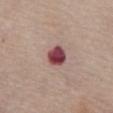Clinical impression: Part of a total-body skin-imaging series; this lesion was reviewed on a skin check and was not flagged for biopsy. Acquisition and patient details: An algorithmic analysis of the crop reported an area of roughly 5.5 mm² and an eccentricity of roughly 0.2. And it measured an average lesion color of about L≈44 a*≈28 b*≈18 (CIELAB), a lesion–skin lightness drop of about 19, and a lesion-to-skin contrast of about 14 (normalized; higher = more distinct). The analysis additionally found a border-irregularity rating of about 1.5/10, a within-lesion color-variation index near 5/10, and peripheral color asymmetry of about 1.5. The software also gave lesion-presence confidence of about 100/100. Measured at roughly 2.5 mm in maximum diameter. A male subject, in their mid-80s or older. This is a white-light tile. This image is a 15 mm lesion crop taken from a total-body photograph. The lesion is located on the abdomen.